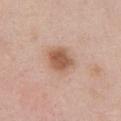Notes:
– workup · no biopsy performed (imaged during a skin exam)
– subject · female, roughly 50 years of age
– location · the abdomen
– image source · ~15 mm crop, total-body skin-cancer survey